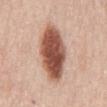Assessment:
Part of a total-body skin-imaging series; this lesion was reviewed on a skin check and was not flagged for biopsy.
Image and clinical context:
The subject is a female aged around 65. The recorded lesion diameter is about 8 mm. A 15 mm close-up extracted from a 3D total-body photography capture. Located on the chest. Imaged with white-light lighting.